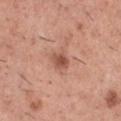Background:
This is a white-light tile. From the chest. A 15 mm close-up extracted from a 3D total-body photography capture. About 2.5 mm across. A male subject aged around 45.